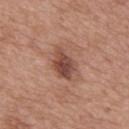Notes:
- notes: imaged on a skin check; not biopsied
- TBP lesion metrics: a classifier nevus-likeness of about 45/100 and lesion-presence confidence of about 100/100
- image source: ~15 mm crop, total-body skin-cancer survey
- anatomic site: the mid back
- patient: male, in their 50s